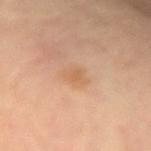This lesion was catalogued during total-body skin photography and was not selected for biopsy. A roughly 15 mm field-of-view crop from a total-body skin photograph. On the left forearm. About 2.5 mm across. The patient is a female aged around 65.